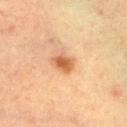• follow-up · no biopsy performed (imaged during a skin exam)
• acquisition · 15 mm crop, total-body photography
• image-analysis metrics · an area of roughly 4.5 mm², an eccentricity of roughly 0.6, and a symmetry-axis asymmetry near 0.2; border irregularity of about 2 on a 0–10 scale and internal color variation of about 3.5 on a 0–10 scale; a classifier nevus-likeness of about 95/100 and a detector confidence of about 100 out of 100 that the crop contains a lesion
• site · the left lower leg
• lighting · cross-polarized illumination
• diameter · ~2.5 mm (longest diameter)
• subject · female, aged around 55